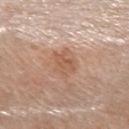biopsy status: catalogued during a skin exam; not biopsied
patient: female, aged 68–72
body site: the left forearm
size: about 4 mm
image source: total-body-photography crop, ~15 mm field of view